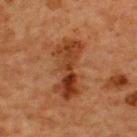Imaged during a routine full-body skin examination; the lesion was not biopsied and no histopathology is available. The lesion's longest dimension is about 7 mm. The lesion-visualizer software estimated a classifier nevus-likeness of about 45/100 and a detector confidence of about 100 out of 100 that the crop contains a lesion. Imaged with cross-polarized lighting. A 15 mm close-up extracted from a 3D total-body photography capture. A male subject, aged 63 to 67. Located on the upper back.